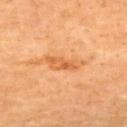Case summary:
* follow-up — no biopsy performed (imaged during a skin exam)
* anatomic site — the upper back
* lighting — cross-polarized
* lesion diameter — about 4.5 mm
* subject — male, aged around 60
* image source — 15 mm crop, total-body photography